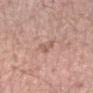Part of a total-body skin-imaging series; this lesion was reviewed on a skin check and was not flagged for biopsy.
The subject is a male aged 48 to 52.
Captured under white-light illumination.
A 15 mm crop from a total-body photograph taken for skin-cancer surveillance.
Longest diameter approximately 2.5 mm.
The lesion is located on the arm.